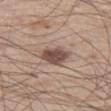acquisition = total-body-photography crop, ~15 mm field of view
tile lighting = white-light
diameter = ≈4 mm
body site = the left thigh
subject = male, aged 58–62
automated lesion analysis = an average lesion color of about L≈50 a*≈17 b*≈24 (CIELAB), a lesion–skin lightness drop of about 14, and a normalized border contrast of about 9.5; a border-irregularity rating of about 2.5/10 and internal color variation of about 3 on a 0–10 scale